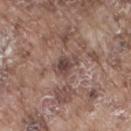Captured during whole-body skin photography for melanoma surveillance; the lesion was not biopsied. The lesion is on the right thigh. Approximately 3 mm at its widest. Cropped from a whole-body photographic skin survey; the tile spans about 15 mm. Captured under white-light illumination. The patient is a male aged around 75.